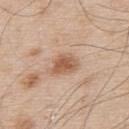Q: Is there a histopathology result?
A: total-body-photography surveillance lesion; no biopsy
Q: Where on the body is the lesion?
A: the upper back
Q: How was the tile lit?
A: white-light illumination
Q: What kind of image is this?
A: ~15 mm crop, total-body skin-cancer survey
Q: Patient demographics?
A: male, aged 53 to 57